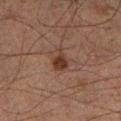Findings:
– follow-up · total-body-photography surveillance lesion; no biopsy
– anatomic site · the left lower leg
– size · ≈3 mm
– tile lighting · cross-polarized illumination
– subject · male, in their 50s
– image-analysis metrics · an eccentricity of roughly 0.7 and a symmetry-axis asymmetry near 0.35; border irregularity of about 3 on a 0–10 scale, a color-variation rating of about 2.5/10, and radial color variation of about 1
– imaging modality · 15 mm crop, total-body photography